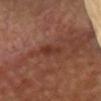size — about 3 mm
image source — 15 mm crop, total-body photography
site — the head or neck
patient — female, aged around 75